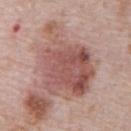Assessment:
This lesion was catalogued during total-body skin photography and was not selected for biopsy.
Image and clinical context:
Measured at roughly 9.5 mm in maximum diameter. A 15 mm close-up tile from a total-body photography series done for melanoma screening. Imaged with white-light lighting. The lesion-visualizer software estimated a shape-asymmetry score of about 0.35 (0 = symmetric). The analysis additionally found a mean CIELAB color near L≈53 a*≈22 b*≈24, a lesion–skin lightness drop of about 12, and a normalized border contrast of about 8. It also reported a border-irregularity index near 4.5/10, internal color variation of about 6.5 on a 0–10 scale, and peripheral color asymmetry of about 2.5. And it measured a classifier nevus-likeness of about 25/100. A male patient approximately 70 years of age. On the front of the torso.